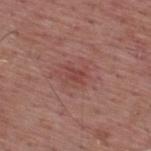| key | value |
|---|---|
| follow-up | no biopsy performed (imaged during a skin exam) |
| lesion size | ≈2.5 mm |
| acquisition | ~15 mm tile from a whole-body skin photo |
| anatomic site | the back |
| patient | male, about 55 years old |
| lighting | white-light illumination |